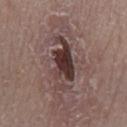| key | value |
|---|---|
| notes | no biopsy performed (imaged during a skin exam) |
| subject | male, aged around 50 |
| imaging modality | ~15 mm tile from a whole-body skin photo |
| illumination | white-light illumination |
| site | the left lower leg |
| automated metrics | an area of roughly 12 mm², an outline eccentricity of about 0.9 (0 = round, 1 = elongated), and a symmetry-axis asymmetry near 0.35 |